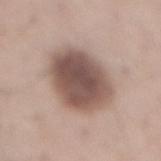On the mid back. About 6.5 mm across. Automated image analysis of the tile measured a lesion area of about 28 mm², an eccentricity of roughly 0.55, and a symmetry-axis asymmetry near 0.1. The analysis additionally found border irregularity of about 1.5 on a 0–10 scale, a color-variation rating of about 5/10, and a peripheral color-asymmetry measure near 1.5. A roughly 15 mm field-of-view crop from a total-body skin photograph. Imaged with white-light lighting. A male patient, aged 53 to 57.